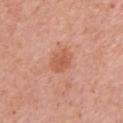From the right upper arm.
A roughly 15 mm field-of-view crop from a total-body skin photograph.
A female patient, aged 63 to 67.